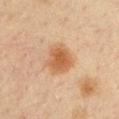Recorded during total-body skin imaging; not selected for excision or biopsy. Captured under cross-polarized illumination. The total-body-photography lesion software estimated an average lesion color of about L≈54 a*≈21 b*≈36 (CIELAB). It also reported internal color variation of about 3 on a 0–10 scale and a peripheral color-asymmetry measure near 1. And it measured an automated nevus-likeness rating near 100 out of 100 and a lesion-detection confidence of about 100/100. The patient is a male aged approximately 35. About 4 mm across. A 15 mm close-up tile from a total-body photography series done for melanoma screening. On the arm.